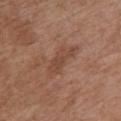Case summary:
* follow-up · total-body-photography surveillance lesion; no biopsy
* imaging modality · ~15 mm crop, total-body skin-cancer survey
* anatomic site · the chest
* patient · female, about 75 years old
* size · ≈5 mm
* TBP lesion metrics · an area of roughly 7 mm², an eccentricity of roughly 0.9, and a symmetry-axis asymmetry near 0.35; border irregularity of about 4 on a 0–10 scale, a within-lesion color-variation index near 2/10, and peripheral color asymmetry of about 1
* lighting · white-light